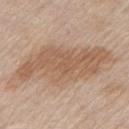Assessment:
The lesion was photographed on a routine skin check and not biopsied; there is no pathology result.
Background:
From the front of the torso. A 15 mm crop from a total-body photograph taken for skin-cancer surveillance. A male subject, aged approximately 80. This is a white-light tile. The lesion's longest dimension is about 11.5 mm.